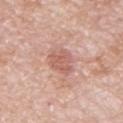patient:
  sex: male
  age_approx: 65
site: abdomen
image:
  source: total-body photography crop
  field_of_view_mm: 15
lesion_size:
  long_diameter_mm_approx: 3.5
automated_metrics:
  area_mm2_approx: 7.0
  eccentricity: 0.7
  shape_asymmetry: 0.25
  cielab_L: 60
  cielab_a: 24
  cielab_b: 27
  vs_skin_darker_L: 9.0
  vs_skin_contrast_norm: 6.0
  nevus_likeness_0_100: 15
  lesion_detection_confidence_0_100: 100